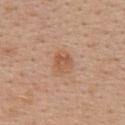workup: imaged on a skin check; not biopsied | imaging modality: 15 mm crop, total-body photography | patient: female, aged 38 to 42 | anatomic site: the upper back | lighting: white-light.